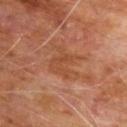Clinical impression:
Recorded during total-body skin imaging; not selected for excision or biopsy.
Context:
A 15 mm close-up extracted from a 3D total-body photography capture. Located on the chest. An algorithmic analysis of the crop reported border irregularity of about 6 on a 0–10 scale, a within-lesion color-variation index near 2.5/10, and peripheral color asymmetry of about 0.5. The patient is a male aged 58 to 62. The recorded lesion diameter is about 4 mm.